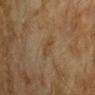Imaged during a routine full-body skin examination; the lesion was not biopsied and no histopathology is available.
The patient is a female aged 53–57.
The lesion is located on the arm.
A lesion tile, about 15 mm wide, cut from a 3D total-body photograph.
Imaged with cross-polarized lighting.
Automated image analysis of the tile measured a mean CIELAB color near L≈38 a*≈14 b*≈29. It also reported a border-irregularity rating of about 3/10, a within-lesion color-variation index near 1.5/10, and peripheral color asymmetry of about 0.5. It also reported a nevus-likeness score of about 0/100 and a lesion-detection confidence of about 100/100.